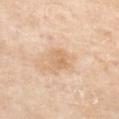Impression: The lesion was photographed on a routine skin check and not biopsied; there is no pathology result. Context: A region of skin cropped from a whole-body photographic capture, roughly 15 mm wide. The tile uses white-light illumination. From the left forearm. Automated tile analysis of the lesion measured a footprint of about 4 mm², a shape eccentricity near 0.75, and a symmetry-axis asymmetry near 0.2. And it measured internal color variation of about 2.5 on a 0–10 scale and a peripheral color-asymmetry measure near 1. The patient is a female aged approximately 70.